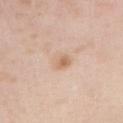follow-up: catalogued during a skin exam; not biopsied
patient: male, aged around 55
diameter: ~3 mm (longest diameter)
illumination: white-light illumination
body site: the chest
imaging modality: ~15 mm tile from a whole-body skin photo